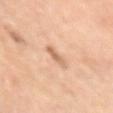The lesion was photographed on a routine skin check and not biopsied; there is no pathology result. Located on the right upper arm. Cropped from a whole-body photographic skin survey; the tile spans about 15 mm. Approximately 2.5 mm at its widest. This is a cross-polarized tile. The patient is a female in their mid-60s.